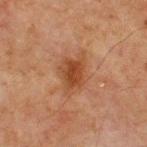Imaged during a routine full-body skin examination; the lesion was not biopsied and no histopathology is available.